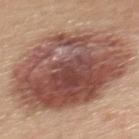workup=catalogued during a skin exam; not biopsied
anatomic site=the upper back
lesion size=≈12 mm
patient=female, roughly 45 years of age
imaging modality=total-body-photography crop, ~15 mm field of view
lighting=white-light